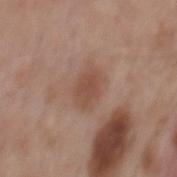No biopsy was performed on this lesion — it was imaged during a full skin examination and was not determined to be concerning. Measured at roughly 3.5 mm in maximum diameter. Automated tile analysis of the lesion measured a shape eccentricity near 0.75 and two-axis asymmetry of about 0.2. On the back. Imaged with white-light lighting. A 15 mm crop from a total-body photograph taken for skin-cancer surveillance. A male patient aged 53 to 57.